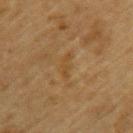biopsy status: catalogued during a skin exam; not biopsied
image: ~15 mm tile from a whole-body skin photo
site: the left upper arm
patient: male, in their mid-80s or older
lesion diameter: about 3 mm
illumination: cross-polarized illumination